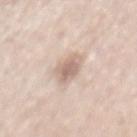Notes:
- biopsy status · total-body-photography surveillance lesion; no biopsy
- anatomic site · the back
- image-analysis metrics · a shape eccentricity near 0.85 and a symmetry-axis asymmetry near 0.2; a color-variation rating of about 3.5/10
- tile lighting · white-light
- imaging modality · 15 mm crop, total-body photography
- subject · male, aged 63–67
- lesion size · ~4 mm (longest diameter)